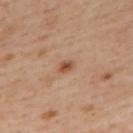Clinical impression: The lesion was tiled from a total-body skin photograph and was not biopsied. Acquisition and patient details: On the upper back. A female patient about 40 years old. About 2 mm across. A 15 mm close-up extracted from a 3D total-body photography capture.